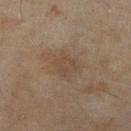Notes:
- biopsy status: total-body-photography surveillance lesion; no biopsy
- lesion diameter: ≈2.5 mm
- illumination: cross-polarized illumination
- subject: female, aged approximately 60
- anatomic site: the right lower leg
- automated metrics: a lesion-detection confidence of about 100/100
- imaging modality: 15 mm crop, total-body photography